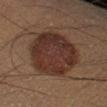Findings:
* workup — imaged on a skin check; not biopsied
* patient — male, aged approximately 40
* image — 15 mm crop, total-body photography
* location — the right lower leg
* tile lighting — cross-polarized
* automated metrics — lesion-presence confidence of about 100/100
* size — ≈7.5 mm